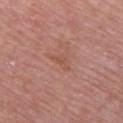Captured during whole-body skin photography for melanoma surveillance; the lesion was not biopsied.
Approximately 2.5 mm at its widest.
Captured under white-light illumination.
A region of skin cropped from a whole-body photographic capture, roughly 15 mm wide.
The lesion is located on the front of the torso.
A female patient, roughly 65 years of age.
Automated tile analysis of the lesion measured a lesion color around L≈52 a*≈24 b*≈29 in CIELAB, about 5 CIELAB-L* units darker than the surrounding skin, and a lesion-to-skin contrast of about 5 (normalized; higher = more distinct). The analysis additionally found a border-irregularity rating of about 5.5/10.